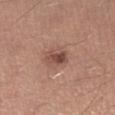Part of a total-body skin-imaging series; this lesion was reviewed on a skin check and was not flagged for biopsy.
An algorithmic analysis of the crop reported a lesion area of about 5 mm², an outline eccentricity of about 0.75 (0 = round, 1 = elongated), and a symmetry-axis asymmetry near 0.2. It also reported an average lesion color of about L≈48 a*≈22 b*≈25 (CIELAB), roughly 11 lightness units darker than nearby skin, and a lesion-to-skin contrast of about 8 (normalized; higher = more distinct). It also reported a within-lesion color-variation index near 4/10 and radial color variation of about 1.5.
A 15 mm crop from a total-body photograph taken for skin-cancer surveillance.
The patient is a male roughly 40 years of age.
The lesion is located on the right lower leg.